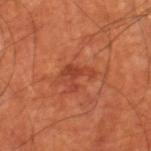The lesion was tiled from a total-body skin photograph and was not biopsied. This image is a 15 mm lesion crop taken from a total-body photograph. From the right thigh. The subject is a male roughly 70 years of age. This is a cross-polarized tile. About 3.5 mm across.